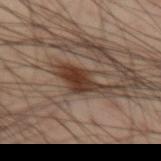The lesion was tiled from a total-body skin photograph and was not biopsied.
Imaged with cross-polarized lighting.
A 15 mm crop from a total-body photograph taken for skin-cancer surveillance.
From the left thigh.
A male subject, in their mid- to late 50s.